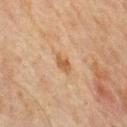  image:
    source: total-body photography crop
    field_of_view_mm: 15
  lesion_size:
    long_diameter_mm_approx: 2.5
  site: mid back
  patient:
    sex: male
    age_approx: 65
  lighting: cross-polarized